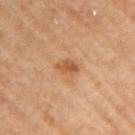Q: Is there a histopathology result?
A: catalogued during a skin exam; not biopsied
Q: Automated lesion metrics?
A: a border-irregularity rating of about 2/10, internal color variation of about 2.5 on a 0–10 scale, and peripheral color asymmetry of about 1; an automated nevus-likeness rating near 45 out of 100 and a detector confidence of about 100 out of 100 that the crop contains a lesion
Q: How was this image acquired?
A: 15 mm crop, total-body photography
Q: Illumination type?
A: cross-polarized illumination
Q: How large is the lesion?
A: ≈3 mm
Q: What is the anatomic site?
A: the left arm
Q: Patient demographics?
A: female, aged 63–67